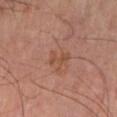{"biopsy_status": "not biopsied; imaged during a skin examination", "patient": {"sex": "male", "age_approx": 60}, "lesion_size": {"long_diameter_mm_approx": 2.5}, "automated_metrics": {"vs_skin_darker_L": 6.0, "vs_skin_contrast_norm": 5.5, "color_variation_0_10": 0.0, "peripheral_color_asymmetry": 0.0}, "site": "left lower leg", "image": {"source": "total-body photography crop", "field_of_view_mm": 15}, "lighting": "cross-polarized"}